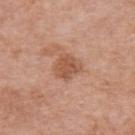{"biopsy_status": "not biopsied; imaged during a skin examination", "lesion_size": {"long_diameter_mm_approx": 3.0}, "patient": {"sex": "female", "age_approx": 70}, "image": {"source": "total-body photography crop", "field_of_view_mm": 15}, "site": "left upper arm", "automated_metrics": {"cielab_L": 54, "cielab_a": 23, "cielab_b": 32, "vs_skin_darker_L": 10.0, "vs_skin_contrast_norm": 7.0, "border_irregularity_0_10": 2.5, "color_variation_0_10": 2.0, "peripheral_color_asymmetry": 0.5, "nevus_likeness_0_100": 10, "lesion_detection_confidence_0_100": 100}}